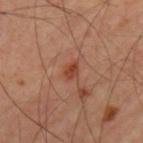The lesion was photographed on a routine skin check and not biopsied; there is no pathology result. Located on the upper back. Cropped from a total-body skin-imaging series; the visible field is about 15 mm. The lesion-visualizer software estimated a mean CIELAB color near L≈43 a*≈25 b*≈33 and roughly 9 lightness units darker than nearby skin. The software also gave border irregularity of about 1.5 on a 0–10 scale, a within-lesion color-variation index near 2/10, and peripheral color asymmetry of about 1. The analysis additionally found a classifier nevus-likeness of about 35/100 and a lesion-detection confidence of about 100/100. A male patient approximately 65 years of age.